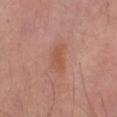| field | value |
|---|---|
| notes | total-body-photography surveillance lesion; no biopsy |
| image-analysis metrics | a lesion color around L≈50 a*≈23 b*≈29 in CIELAB, roughly 7 lightness units darker than nearby skin, and a lesion-to-skin contrast of about 6.5 (normalized; higher = more distinct); border irregularity of about 4 on a 0–10 scale and a within-lesion color-variation index near 2/10 |
| image source | total-body-photography crop, ~15 mm field of view |
| patient | male, aged around 65 |
| lesion size | ≈3.5 mm |
| location | the left thigh |
| lighting | cross-polarized |